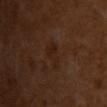Captured during whole-body skin photography for melanoma surveillance; the lesion was not biopsied.
A female subject in their mid-50s.
A lesion tile, about 15 mm wide, cut from a 3D total-body photograph.
From the chest.
Imaged with cross-polarized lighting.